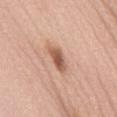<record>
<biopsy_status>not biopsied; imaged during a skin examination</biopsy_status>
<site>mid back</site>
<image>
  <source>total-body photography crop</source>
  <field_of_view_mm>15</field_of_view_mm>
</image>
<patient>
  <sex>female</sex>
  <age_approx>60</age_approx>
</patient>
</record>